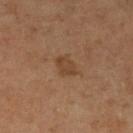No biopsy was performed on this lesion — it was imaged during a full skin examination and was not determined to be concerning.
The lesion is on the leg.
Longest diameter approximately 2.5 mm.
A female patient aged 63 to 67.
Cropped from a whole-body photographic skin survey; the tile spans about 15 mm.